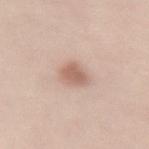notes — catalogued during a skin exam; not biopsied
automated metrics — an area of roughly 5 mm², an eccentricity of roughly 0.7, and two-axis asymmetry of about 0.25; border irregularity of about 2 on a 0–10 scale, a within-lesion color-variation index near 1.5/10, and radial color variation of about 0.5; lesion-presence confidence of about 100/100
subject — female, roughly 40 years of age
lighting — white-light illumination
acquisition — ~15 mm crop, total-body skin-cancer survey
site — the lower back